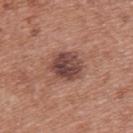Impression: The lesion was tiled from a total-body skin photograph and was not biopsied. Image and clinical context: The lesion-visualizer software estimated a lesion color around L≈44 a*≈22 b*≈23 in CIELAB and roughly 13 lightness units darker than nearby skin. From the upper back. Cropped from a total-body skin-imaging series; the visible field is about 15 mm. A female patient aged 38–42.